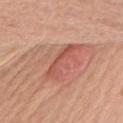The lesion was tiled from a total-body skin photograph and was not biopsied.
About 5 mm across.
The patient is a female in their 50s.
Captured under white-light illumination.
Cropped from a total-body skin-imaging series; the visible field is about 15 mm.
Automated tile analysis of the lesion measured a border-irregularity rating of about 7.5/10 and a color-variation rating of about 4.5/10. It also reported a classifier nevus-likeness of about 70/100 and a detector confidence of about 60 out of 100 that the crop contains a lesion.
From the left upper arm.